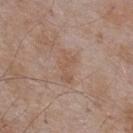notes: total-body-photography surveillance lesion; no biopsy.